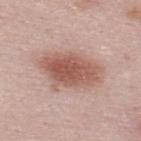  biopsy_status: not biopsied; imaged during a skin examination
  site: upper back
  patient:
    sex: male
    age_approx: 25
  image:
    source: total-body photography crop
    field_of_view_mm: 15
  lesion_size:
    long_diameter_mm_approx: 7.0
  lighting: white-light
  automated_metrics:
    area_mm2_approx: 22.0
    eccentricity: 0.8
    nevus_likeness_0_100: 100